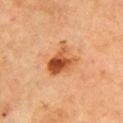Clinical impression: The lesion was tiled from a total-body skin photograph and was not biopsied. Acquisition and patient details: The lesion is on the arm. A female subject aged around 70. Captured under cross-polarized illumination. A close-up tile cropped from a whole-body skin photograph, about 15 mm across. The lesion's longest dimension is about 4.5 mm.